A 15 mm close-up extracted from a 3D total-body photography capture. Located on the chest. The patient is a male aged 58–62: 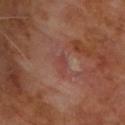automated lesion analysis = a classifier nevus-likeness of about 0/100 and lesion-presence confidence of about 95/100
lighting = cross-polarized
pathology = a squamous cell carcinoma in situ